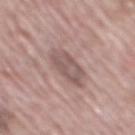Part of a total-body skin-imaging series; this lesion was reviewed on a skin check and was not flagged for biopsy. Automated image analysis of the tile measured an average lesion color of about L≈54 a*≈17 b*≈20 (CIELAB) and roughly 10 lightness units darker than nearby skin. It also reported a classifier nevus-likeness of about 0/100 and a detector confidence of about 90 out of 100 that the crop contains a lesion. The lesion is located on the mid back. A male subject about 70 years old. Approximately 5 mm at its widest. This image is a 15 mm lesion crop taken from a total-body photograph.